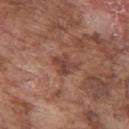{"biopsy_status": "not biopsied; imaged during a skin examination"}